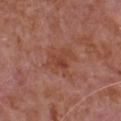notes — imaged on a skin check; not biopsied | image source — ~15 mm crop, total-body skin-cancer survey | automated lesion analysis — about 6 CIELAB-L* units darker than the surrounding skin and a lesion-to-skin contrast of about 6.5 (normalized; higher = more distinct) | illumination — white-light | size — ~4 mm (longest diameter) | subject — male, aged 63–67 | anatomic site — the chest.